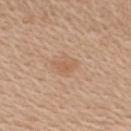A region of skin cropped from a whole-body photographic capture, roughly 15 mm wide.
The lesion's longest dimension is about 2.5 mm.
Imaged with white-light lighting.
A male patient, aged 58–62.
From the right upper arm.
An algorithmic analysis of the crop reported an average lesion color of about L≈59 a*≈20 b*≈33 (CIELAB) and roughly 6 lightness units darker than nearby skin. And it measured a border-irregularity rating of about 4/10 and radial color variation of about 0.5.